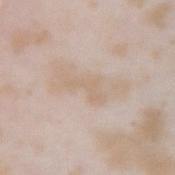No biopsy was performed on this lesion — it was imaged during a full skin examination and was not determined to be concerning.